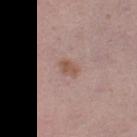The lesion was photographed on a routine skin check and not biopsied; there is no pathology result.
A region of skin cropped from a whole-body photographic capture, roughly 15 mm wide.
The tile uses white-light illumination.
The lesion is on the leg.
Longest diameter approximately 2.5 mm.
The subject is a female aged approximately 20.
Automated image analysis of the tile measured an average lesion color of about L≈53 a*≈19 b*≈26 (CIELAB), roughly 9 lightness units darker than nearby skin, and a lesion-to-skin contrast of about 7.5 (normalized; higher = more distinct).